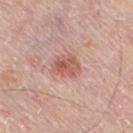No biopsy was performed on this lesion — it was imaged during a full skin examination and was not determined to be concerning.
A 15 mm crop from a total-body photograph taken for skin-cancer surveillance.
On the right thigh.
The tile uses white-light illumination.
The subject is a male aged 78 to 82.